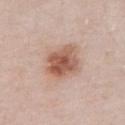Impression: Captured during whole-body skin photography for melanoma surveillance; the lesion was not biopsied. Acquisition and patient details: A male subject in their mid- to late 50s. A close-up tile cropped from a whole-body skin photograph, about 15 mm across. Captured under white-light illumination. Located on the abdomen. Longest diameter approximately 4.5 mm.